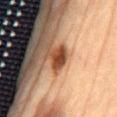<case>
<biopsy_status>not biopsied; imaged during a skin examination</biopsy_status>
<lighting>cross-polarized</lighting>
<image>
  <source>total-body photography crop</source>
  <field_of_view_mm>15</field_of_view_mm>
</image>
<automated_metrics>
  <border_irregularity_0_10>2.5</border_irregularity_0_10>
  <color_variation_0_10>3.0</color_variation_0_10>
  <peripheral_color_asymmetry>1.0</peripheral_color_asymmetry>
  <nevus_likeness_0_100>95</nevus_likeness_0_100>
  <lesion_detection_confidence_0_100>100</lesion_detection_confidence_0_100>
</automated_metrics>
<site>abdomen</site>
<lesion_size>
  <long_diameter_mm_approx>3.0</long_diameter_mm_approx>
</lesion_size>
<patient>
  <sex>male</sex>
  <age_approx>70</age_approx>
</patient>
</case>